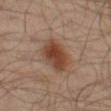workup: imaged on a skin check; not biopsied
image-analysis metrics: a normalized border contrast of about 9.5; a detector confidence of about 100 out of 100 that the crop contains a lesion
patient: male, aged approximately 65
location: the left thigh
imaging modality: ~15 mm tile from a whole-body skin photo